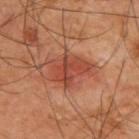{"biopsy_status": "not biopsied; imaged during a skin examination", "image": {"source": "total-body photography crop", "field_of_view_mm": 15}, "patient": {"sex": "male", "age_approx": 60}, "lighting": "cross-polarized", "site": "back", "automated_metrics": {"area_mm2_approx": 11.0, "shape_asymmetry": 0.35, "cielab_L": 44, "cielab_a": 29, "cielab_b": 31, "vs_skin_darker_L": 10.0, "vs_skin_contrast_norm": 7.5, "nevus_likeness_0_100": 90, "lesion_detection_confidence_0_100": 100}}